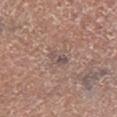Impression:
The lesion was tiled from a total-body skin photograph and was not biopsied.
Context:
From the leg. Captured under white-light illumination. A 15 mm crop from a total-body photograph taken for skin-cancer surveillance. A male patient roughly 80 years of age.